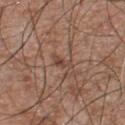The lesion's longest dimension is about 2.5 mm. The patient is a male in their mid- to late 50s. The lesion is located on the chest. A roughly 15 mm field-of-view crop from a total-body skin photograph. The tile uses white-light illumination.